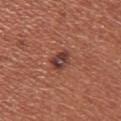Notes:
• site — the chest
• patient — female, aged 33 to 37
• image source — 15 mm crop, total-body photography